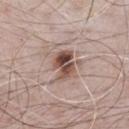workup: catalogued during a skin exam; not biopsied
anatomic site: the chest
subject: male, aged approximately 65
image: 15 mm crop, total-body photography